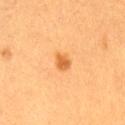Notes:
* workup — no biopsy performed (imaged during a skin exam)
* site — the lower back
* automated lesion analysis — a mean CIELAB color near L≈52 a*≈24 b*≈42, roughly 10 lightness units darker than nearby skin, and a normalized border contrast of about 7.5
* patient — female, aged approximately 40
* image — total-body-photography crop, ~15 mm field of view
* lighting — cross-polarized